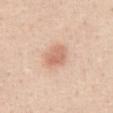Part of a total-body skin-imaging series; this lesion was reviewed on a skin check and was not flagged for biopsy. A female subject, roughly 45 years of age. Approximately 3.5 mm at its widest. The lesion-visualizer software estimated a footprint of about 7 mm², an eccentricity of roughly 0.65, and a shape-asymmetry score of about 0.2 (0 = symmetric). It also reported an automated nevus-likeness rating near 95 out of 100 and a detector confidence of about 100 out of 100 that the crop contains a lesion. From the abdomen. This image is a 15 mm lesion crop taken from a total-body photograph.